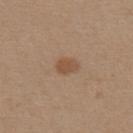No biopsy was performed on this lesion — it was imaged during a full skin examination and was not determined to be concerning. The lesion is on the upper back. A close-up tile cropped from a whole-body skin photograph, about 15 mm across. A female patient, aged 28–32.